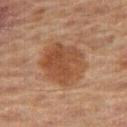site: the leg | tile lighting: cross-polarized | diameter: ≈5.5 mm | subject: male, aged approximately 65 | imaging modality: ~15 mm crop, total-body skin-cancer survey.